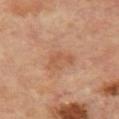Part of a total-body skin-imaging series; this lesion was reviewed on a skin check and was not flagged for biopsy. On the chest. A close-up tile cropped from a whole-body skin photograph, about 15 mm across. A male patient about 70 years old.